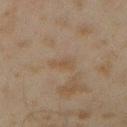Clinical impression:
This lesion was catalogued during total-body skin photography and was not selected for biopsy.
Image and clinical context:
A male patient, aged around 45. A lesion tile, about 15 mm wide, cut from a 3D total-body photograph. Located on the right forearm. The tile uses cross-polarized illumination.